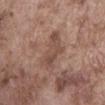The lesion is located on the abdomen.
A 15 mm close-up tile from a total-body photography series done for melanoma screening.
The patient is a male in their mid-70s.